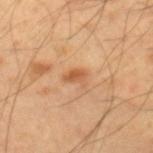biopsy status — total-body-photography surveillance lesion; no biopsy | tile lighting — cross-polarized illumination | anatomic site — the right lower leg | patient — male, about 40 years old | image — 15 mm crop, total-body photography | image-analysis metrics — a lesion area of about 3 mm², a shape eccentricity near 0.75, and a shape-asymmetry score of about 0.4 (0 = symmetric); an automated nevus-likeness rating near 0 out of 100 and a lesion-detection confidence of about 100/100 | lesion diameter — about 2.5 mm.